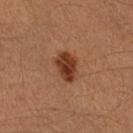- notes: catalogued during a skin exam; not biopsied
- site: the right lower leg
- patient: male, about 40 years old
- imaging modality: ~15 mm crop, total-body skin-cancer survey